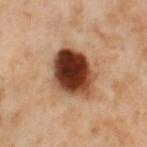The lesion is on the left thigh. A 15 mm close-up extracted from a 3D total-body photography capture. A female subject, aged 53 to 57. Measured at roughly 6 mm in maximum diameter.